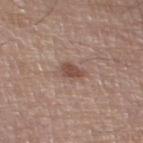<record>
<biopsy_status>not biopsied; imaged during a skin examination</biopsy_status>
<patient>
  <sex>male</sex>
  <age_approx>65</age_approx>
</patient>
<site>left thigh</site>
<lighting>white-light</lighting>
<automated_metrics>
  <area_mm2_approx>3.5</area_mm2_approx>
  <shape_asymmetry>0.25</shape_asymmetry>
  <border_irregularity_0_10>2.0</border_irregularity_0_10>
  <color_variation_0_10>1.5</color_variation_0_10>
  <peripheral_color_asymmetry>0.5</peripheral_color_asymmetry>
  <nevus_likeness_0_100>60</nevus_likeness_0_100>
</automated_metrics>
<image>
  <source>total-body photography crop</source>
  <field_of_view_mm>15</field_of_view_mm>
</image>
<lesion_size>
  <long_diameter_mm_approx>2.5</long_diameter_mm_approx>
</lesion_size>
</record>